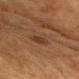notes = catalogued during a skin exam; not biopsied | image = 15 mm crop, total-body photography | diameter = about 3.5 mm | tile lighting = cross-polarized illumination | TBP lesion metrics = a lesion area of about 5.5 mm²; a lesion color around L≈30 a*≈16 b*≈26 in CIELAB, roughly 5 lightness units darker than nearby skin, and a normalized border contrast of about 5.5; border irregularity of about 3.5 on a 0–10 scale and a peripheral color-asymmetry measure near 0.5 | site = the chest | subject = male, in their mid-70s.